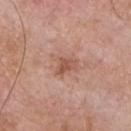Case summary:
• workup — no biopsy performed (imaged during a skin exam)
• acquisition — total-body-photography crop, ~15 mm field of view
• subject — male, aged approximately 60
• location — the chest